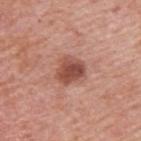On the upper back.
Longest diameter approximately 4 mm.
A lesion tile, about 15 mm wide, cut from a 3D total-body photograph.
A male subject, in their mid- to late 70s.